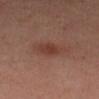– follow-up: catalogued during a skin exam; not biopsied
– image: 15 mm crop, total-body photography
– body site: the left upper arm
– image-analysis metrics: a lesion area of about 6 mm², an eccentricity of roughly 0.5, and two-axis asymmetry of about 0.15; an average lesion color of about L≈38 a*≈22 b*≈26 (CIELAB), a lesion–skin lightness drop of about 7, and a normalized border contrast of about 6.5; lesion-presence confidence of about 100/100
– illumination: cross-polarized illumination
– subject: female, approximately 40 years of age